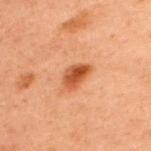Impression:
The lesion was tiled from a total-body skin photograph and was not biopsied.
Context:
From the upper back. The patient is a male aged 58 to 62. A roughly 15 mm field-of-view crop from a total-body skin photograph.